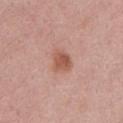Impression:
Imaged during a routine full-body skin examination; the lesion was not biopsied and no histopathology is available.
Clinical summary:
The lesion's longest dimension is about 2.5 mm. The subject is a male roughly 60 years of age. Captured under white-light illumination. This image is a 15 mm lesion crop taken from a total-body photograph. The lesion is on the chest. Automated tile analysis of the lesion measured a mean CIELAB color near L≈55 a*≈24 b*≈29 and a lesion–skin lightness drop of about 10. It also reported a classifier nevus-likeness of about 90/100 and lesion-presence confidence of about 100/100.